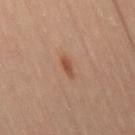{
  "biopsy_status": "not biopsied; imaged during a skin examination",
  "image": {
    "source": "total-body photography crop",
    "field_of_view_mm": 15
  },
  "lesion_size": {
    "long_diameter_mm_approx": 2.5
  },
  "site": "leg",
  "patient": {
    "sex": "female",
    "age_approx": 40
  },
  "automated_metrics": {
    "vs_skin_darker_L": 10.0,
    "vs_skin_contrast_norm": 7.5,
    "border_irregularity_0_10": 3.0,
    "color_variation_0_10": 0.0,
    "peripheral_color_asymmetry": 0.0,
    "nevus_likeness_0_100": 70
  },
  "lighting": "cross-polarized"
}